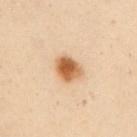Q: Is there a histopathology result?
A: total-body-photography surveillance lesion; no biopsy
Q: Who is the patient?
A: female, about 50 years old
Q: How was this image acquired?
A: 15 mm crop, total-body photography
Q: What is the lesion's diameter?
A: about 3 mm
Q: Lesion location?
A: the abdomen
Q: What did automated image analysis measure?
A: an area of roughly 7 mm² and two-axis asymmetry of about 0.2; border irregularity of about 1.5 on a 0–10 scale, a within-lesion color-variation index near 5.5/10, and peripheral color asymmetry of about 1.5